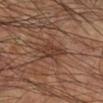  biopsy_status: not biopsied; imaged during a skin examination
  site: left upper arm
  lighting: cross-polarized
  patient:
    sex: male
    age_approx: 75
  image:
    source: total-body photography crop
    field_of_view_mm: 15
  automated_metrics:
    eccentricity: 0.65
    color_variation_0_10: 2.0
    peripheral_color_asymmetry: 0.5
    nevus_likeness_0_100: 5
  lesion_size:
    long_diameter_mm_approx: 3.0